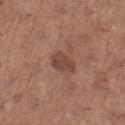Captured during whole-body skin photography for melanoma surveillance; the lesion was not biopsied. A female patient, in their mid- to late 60s. From the right lower leg. A region of skin cropped from a whole-body photographic capture, roughly 15 mm wide. Imaged with white-light lighting. Approximately 3.5 mm at its widest.